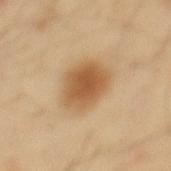Captured during whole-body skin photography for melanoma surveillance; the lesion was not biopsied.
A 15 mm close-up extracted from a 3D total-body photography capture.
Measured at roughly 5 mm in maximum diameter.
On the mid back.
A male patient, in their 40s.